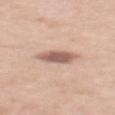• illumination · white-light
• imaging modality · ~15 mm crop, total-body skin-cancer survey
• site · the upper back
• patient · female, aged 53–57